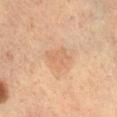| field | value |
|---|---|
| workup | total-body-photography surveillance lesion; no biopsy |
| site | the leg |
| image | ~15 mm tile from a whole-body skin photo |
| patient | female, approximately 55 years of age |